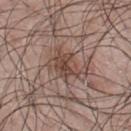notes: imaged on a skin check; not biopsied
illumination: white-light
location: the upper back
subject: male, aged 43 to 47
acquisition: ~15 mm tile from a whole-body skin photo